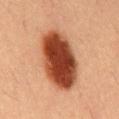notes: imaged on a skin check; not biopsied
subject: male, aged around 55
tile lighting: cross-polarized illumination
location: the chest
automated lesion analysis: a lesion area of about 29 mm², an outline eccentricity of about 0.8 (0 = round, 1 = elongated), and two-axis asymmetry of about 0.1; a mean CIELAB color near L≈39 a*≈26 b*≈32, a lesion–skin lightness drop of about 20, and a normalized border contrast of about 14.5; a within-lesion color-variation index near 7.5/10 and a peripheral color-asymmetry measure near 2.5
image: ~15 mm crop, total-body skin-cancer survey
diameter: about 8 mm